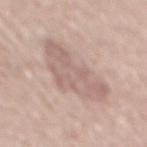patient = male, in their mid- to late 50s
body site = the mid back
acquisition = 15 mm crop, total-body photography
histopathologic diagnosis = a dysplastic (Clark) nevus — a benign lesion